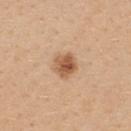biopsy_status: not biopsied; imaged during a skin examination
image:
  source: total-body photography crop
  field_of_view_mm: 15
lighting: white-light
patient:
  sex: female
  age_approx: 25
automated_metrics:
  cielab_L: 56
  cielab_a: 21
  cielab_b: 35
  vs_skin_darker_L: 13.0
  vs_skin_contrast_norm: 9.0
site: back